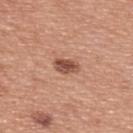{"lesion_size": {"long_diameter_mm_approx": 3.0}, "site": "upper back", "image": {"source": "total-body photography crop", "field_of_view_mm": 15}, "patient": {"sex": "male", "age_approx": 50}, "automated_metrics": {"border_irregularity_0_10": 2.0, "color_variation_0_10": 3.5, "peripheral_color_asymmetry": 1.0}, "lighting": "white-light"}